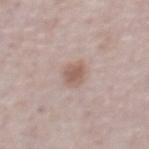The lesion was tiled from a total-body skin photograph and was not biopsied.
Captured under white-light illumination.
An algorithmic analysis of the crop reported a lesion area of about 5.5 mm², a shape eccentricity near 0.6, and two-axis asymmetry of about 0.15. The software also gave a lesion color around L≈58 a*≈17 b*≈24 in CIELAB, roughly 9 lightness units darker than nearby skin, and a normalized border contrast of about 6.5. The analysis additionally found a border-irregularity index near 1.5/10, internal color variation of about 3 on a 0–10 scale, and peripheral color asymmetry of about 1. And it measured an automated nevus-likeness rating near 85 out of 100.
A lesion tile, about 15 mm wide, cut from a 3D total-body photograph.
A male subject aged 73 to 77.
The lesion is on the abdomen.
The lesion's longest dimension is about 3 mm.